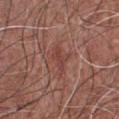biopsy_status: not biopsied; imaged during a skin examination
image:
  source: total-body photography crop
  field_of_view_mm: 15
lesion_size:
  long_diameter_mm_approx: 3.5
patient:
  sex: male
  age_approx: 50
lighting: white-light
site: chest
automated_metrics:
  cielab_L: 43
  cielab_a: 25
  cielab_b: 25
  vs_skin_darker_L: 7.0
  vs_skin_contrast_norm: 5.5
  border_irregularity_0_10: 5.5
  color_variation_0_10: 1.0
  peripheral_color_asymmetry: 0.0
  nevus_likeness_0_100: 0
  lesion_detection_confidence_0_100: 85